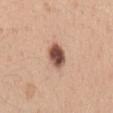<lesion>
  <biopsy_status>not biopsied; imaged during a skin examination</biopsy_status>
  <lighting>white-light</lighting>
  <image>
    <source>total-body photography crop</source>
    <field_of_view_mm>15</field_of_view_mm>
  </image>
  <lesion_size>
    <long_diameter_mm_approx>3.0</long_diameter_mm_approx>
  </lesion_size>
  <site>left upper arm</site>
  <patient>
    <sex>female</sex>
    <age_approx>25</age_approx>
  </patient>
</lesion>